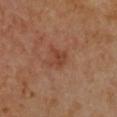Q: What is the imaging modality?
A: 15 mm crop, total-body photography
Q: What are the patient's age and sex?
A: female, aged 53–57
Q: Where on the body is the lesion?
A: the chest
Q: What is the histopathologic diagnosis?
A: a nodular basal cell carcinoma